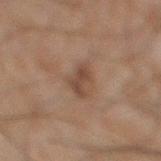Assessment:
Part of a total-body skin-imaging series; this lesion was reviewed on a skin check and was not flagged for biopsy.
Context:
Located on the right lower leg. A male patient aged 43–47. A lesion tile, about 15 mm wide, cut from a 3D total-body photograph.